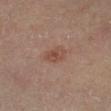{"biopsy_status": "not biopsied; imaged during a skin examination", "lighting": "cross-polarized", "lesion_size": {"long_diameter_mm_approx": 3.0}, "patient": {"sex": "male", "age_approx": 55}, "site": "leg", "image": {"source": "total-body photography crop", "field_of_view_mm": 15}}